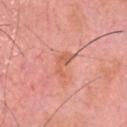Notes:
- workup — imaged on a skin check; not biopsied
- subject — male, in their 80s
- lesion diameter — ~3 mm (longest diameter)
- tile lighting — white-light illumination
- imaging modality — ~15 mm tile from a whole-body skin photo
- body site — the head or neck